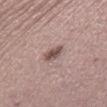biopsy_status: not biopsied; imaged during a skin examination
lighting: white-light
site: right lower leg
patient:
  sex: female
  age_approx: 50
automated_metrics:
  area_mm2_approx: 4.0
  shape_asymmetry: 0.15
  border_irregularity_0_10: 1.5
  color_variation_0_10: 4.5
  nevus_likeness_0_100: 80
image:
  source: total-body photography crop
  field_of_view_mm: 15